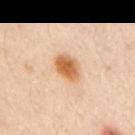follow-up — imaged on a skin check; not biopsied | anatomic site — the right upper arm | subject — male, roughly 45 years of age | image source — ~15 mm tile from a whole-body skin photo | illumination — cross-polarized.